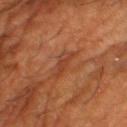Clinical impression: Part of a total-body skin-imaging series; this lesion was reviewed on a skin check and was not flagged for biopsy. Background: A male patient approximately 60 years of age. The lesion's longest dimension is about 3.5 mm. An algorithmic analysis of the crop reported an average lesion color of about L≈40 a*≈27 b*≈33 (CIELAB). This image is a 15 mm lesion crop taken from a total-body photograph. From the right thigh.